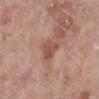{"biopsy_status": "not biopsied; imaged during a skin examination", "patient": {"sex": "female", "age_approx": 70}, "lesion_size": {"long_diameter_mm_approx": 3.0}, "image": {"source": "total-body photography crop", "field_of_view_mm": 15}, "site": "left lower leg", "automated_metrics": {"cielab_L": 51, "cielab_a": 23, "cielab_b": 26, "vs_skin_contrast_norm": 7.0, "nevus_likeness_0_100": 0, "lesion_detection_confidence_0_100": 100}, "lighting": "white-light"}